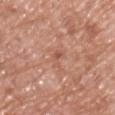<case>
  <biopsy_status>not biopsied; imaged during a skin examination</biopsy_status>
</case>